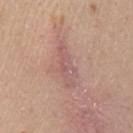Clinical impression:
The lesion was photographed on a routine skin check and not biopsied; there is no pathology result.
Image and clinical context:
A close-up tile cropped from a whole-body skin photograph, about 15 mm across. On the left upper arm. Automated tile analysis of the lesion measured a lesion area of about 7.5 mm², a shape eccentricity near 0.95, and two-axis asymmetry of about 0.3. The analysis additionally found a lesion color around L≈57 a*≈22 b*≈22 in CIELAB, roughly 8 lightness units darker than nearby skin, and a normalized lesion–skin contrast near 6. The software also gave a border-irregularity rating of about 5/10, internal color variation of about 2 on a 0–10 scale, and peripheral color asymmetry of about 0.5. The analysis additionally found lesion-presence confidence of about 15/100. The patient is a female aged approximately 45.